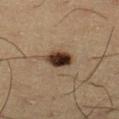Assessment: Captured during whole-body skin photography for melanoma surveillance; the lesion was not biopsied. Image and clinical context: Cropped from a whole-body photographic skin survey; the tile spans about 15 mm. On the leg. The lesion-visualizer software estimated an outline eccentricity of about 0.6 (0 = round, 1 = elongated) and two-axis asymmetry of about 0.15. And it measured a border-irregularity rating of about 1.5/10, a color-variation rating of about 8/10, and peripheral color asymmetry of about 2.5. The analysis additionally found a classifier nevus-likeness of about 100/100 and a detector confidence of about 100 out of 100 that the crop contains a lesion. The subject is a male aged 33–37.